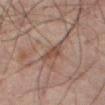biopsy status=imaged on a skin check; not biopsied | size=~3 mm (longest diameter) | image=total-body-photography crop, ~15 mm field of view | patient=male, approximately 65 years of age | illumination=cross-polarized illumination | site=the mid back.